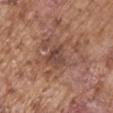follow-up: no biopsy performed (imaged during a skin exam) | subject: male, approximately 75 years of age | tile lighting: white-light illumination | TBP lesion metrics: a lesion color around L≈43 a*≈20 b*≈25 in CIELAB, roughly 8 lightness units darker than nearby skin, and a lesion-to-skin contrast of about 7 (normalized; higher = more distinct); lesion-presence confidence of about 100/100 | image: ~15 mm tile from a whole-body skin photo | location: the right upper arm | lesion size: about 3 mm.